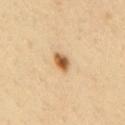notes: imaged on a skin check; not biopsied
body site: the upper back
subject: male, aged approximately 40
TBP lesion metrics: a lesion color around L≈59 a*≈20 b*≈40 in CIELAB, about 16 CIELAB-L* units darker than the surrounding skin, and a normalized border contrast of about 10.5; a color-variation rating of about 5/10 and peripheral color asymmetry of about 1.5
image source: 15 mm crop, total-body photography
illumination: cross-polarized illumination